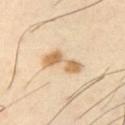Assessment: Part of a total-body skin-imaging series; this lesion was reviewed on a skin check and was not flagged for biopsy. Context: A roughly 15 mm field-of-view crop from a total-body skin photograph. On the left upper arm. A male patient, approximately 40 years of age. Captured under cross-polarized illumination.